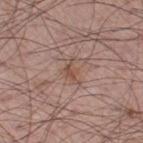Findings:
– site: the left thigh
– lesion diameter: about 3.5 mm
– tile lighting: white-light illumination
– image-analysis metrics: a nevus-likeness score of about 5/100 and a detector confidence of about 100 out of 100 that the crop contains a lesion
– subject: male, roughly 60 years of age
– image source: total-body-photography crop, ~15 mm field of view